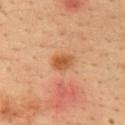Case summary:
– notes · imaged on a skin check; not biopsied
– subject · male, aged 33 to 37
– tile lighting · cross-polarized illumination
– lesion diameter · ≈2.5 mm
– acquisition · ~15 mm crop, total-body skin-cancer survey
– body site · the upper back